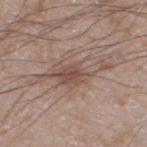Notes:
• follow-up: catalogued during a skin exam; not biopsied
• size: ≈8 mm
• site: the right thigh
• image: ~15 mm tile from a whole-body skin photo
• patient: male, aged around 60